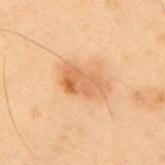Clinical impression:
Recorded during total-body skin imaging; not selected for excision or biopsy.
Image and clinical context:
A 15 mm close-up extracted from a 3D total-body photography capture. A male patient aged 53–57. The lesion is on the mid back. Automated tile analysis of the lesion measured a border-irregularity index near 2.5/10 and a peripheral color-asymmetry measure near 1.5. Imaged with cross-polarized lighting.